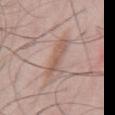The lesion was photographed on a routine skin check and not biopsied; there is no pathology result. The patient is a male about 45 years old. The lesion is located on the mid back. A 15 mm crop from a total-body photograph taken for skin-cancer surveillance.